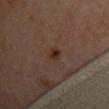<record>
<patient>
  <sex>female</sex>
  <age_approx>60</age_approx>
</patient>
<site>chest</site>
<automated_metrics>
  <cielab_L>31</cielab_L>
  <cielab_a>17</cielab_a>
  <cielab_b>25</cielab_b>
  <vs_skin_darker_L>7.0</vs_skin_darker_L>
  <vs_skin_contrast_norm>8.0</vs_skin_contrast_norm>
  <nevus_likeness_0_100>85</nevus_likeness_0_100>
  <lesion_detection_confidence_0_100>100</lesion_detection_confidence_0_100>
</automated_metrics>
<lesion_size>
  <long_diameter_mm_approx>2.5</long_diameter_mm_approx>
</lesion_size>
<image>
  <source>total-body photography crop</source>
  <field_of_view_mm>15</field_of_view_mm>
</image>
<lighting>cross-polarized</lighting>
</record>